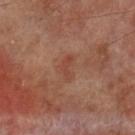Q: Is there a histopathology result?
A: imaged on a skin check; not biopsied
Q: How was the tile lit?
A: cross-polarized
Q: What is the imaging modality?
A: ~15 mm crop, total-body skin-cancer survey
Q: Who is the patient?
A: male, in their 70s
Q: Lesion location?
A: the leg
Q: How large is the lesion?
A: ≈3 mm